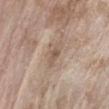This lesion was catalogued during total-body skin photography and was not selected for biopsy.
The tile uses white-light illumination.
From the left forearm.
The patient is a female roughly 75 years of age.
This image is a 15 mm lesion crop taken from a total-body photograph.
About 3 mm across.